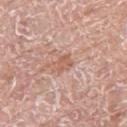Impression:
Part of a total-body skin-imaging series; this lesion was reviewed on a skin check and was not flagged for biopsy.
Image and clinical context:
Automated image analysis of the tile measured an outline eccentricity of about 0.7 (0 = round, 1 = elongated) and a symmetry-axis asymmetry near 0.25. The software also gave a border-irregularity index near 2.5/10, a within-lesion color-variation index near 1.5/10, and a peripheral color-asymmetry measure near 0.5. The lesion is located on the right thigh. A roughly 15 mm field-of-view crop from a total-body skin photograph. The tile uses white-light illumination. A male subject, in their mid- to late 70s.